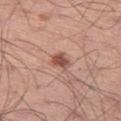Clinical impression: No biopsy was performed on this lesion — it was imaged during a full skin examination and was not determined to be concerning. Background: Longest diameter approximately 2.5 mm. A lesion tile, about 15 mm wide, cut from a 3D total-body photograph. The subject is a male aged 53 to 57. Captured under white-light illumination. From the left thigh. Automated tile analysis of the lesion measured a color-variation rating of about 3/10 and radial color variation of about 1. The analysis additionally found a classifier nevus-likeness of about 90/100 and a lesion-detection confidence of about 100/100.